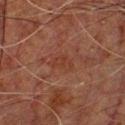Recorded during total-body skin imaging; not selected for excision or biopsy.
Longest diameter approximately 3 mm.
From the chest.
A male patient roughly 50 years of age.
Cropped from a whole-body photographic skin survey; the tile spans about 15 mm.
An algorithmic analysis of the crop reported an average lesion color of about L≈29 a*≈20 b*≈24 (CIELAB) and a lesion–skin lightness drop of about 4.
Imaged with cross-polarized lighting.